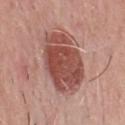Assessment:
The lesion was photographed on a routine skin check and not biopsied; there is no pathology result.
Background:
The tile uses white-light illumination. On the chest. The lesion's longest dimension is about 8 mm. A male subject aged 48 to 52. Cropped from a total-body skin-imaging series; the visible field is about 15 mm.